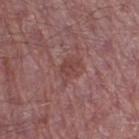<record>
  <biopsy_status>not biopsied; imaged during a skin examination</biopsy_status>
  <image>
    <source>total-body photography crop</source>
    <field_of_view_mm>15</field_of_view_mm>
  </image>
  <lighting>white-light</lighting>
  <patient>
    <sex>male</sex>
    <age_approx>65</age_approx>
  </patient>
  <lesion_size>
    <long_diameter_mm_approx>3.5</long_diameter_mm_approx>
  </lesion_size>
  <site>right thigh</site>
</record>